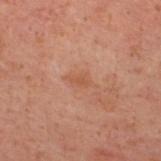{
  "biopsy_status": "not biopsied; imaged during a skin examination",
  "site": "upper back",
  "patient": {
    "sex": "male",
    "age_approx": 40
  },
  "image": {
    "source": "total-body photography crop",
    "field_of_view_mm": 15
  },
  "lighting": "cross-polarized"
}